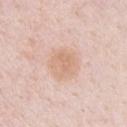{
  "site": "chest",
  "patient": {
    "sex": "male",
    "age_approx": 35
  },
  "image": {
    "source": "total-body photography crop",
    "field_of_view_mm": 15
  },
  "automated_metrics": {
    "border_irregularity_0_10": 3.0,
    "peripheral_color_asymmetry": 0.5
  },
  "lighting": "white-light",
  "lesion_size": {
    "long_diameter_mm_approx": 3.5
  }
}